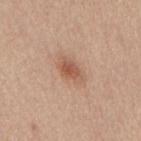| key | value |
|---|---|
| follow-up | imaged on a skin check; not biopsied |
| image source | total-body-photography crop, ~15 mm field of view |
| body site | the mid back |
| subject | female, aged 53 to 57 |